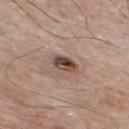<record>
<biopsy_status>not biopsied; imaged during a skin examination</biopsy_status>
<patient>
  <sex>male</sex>
  <age_approx>80</age_approx>
</patient>
<image>
  <source>total-body photography crop</source>
  <field_of_view_mm>15</field_of_view_mm>
</image>
<lighting>white-light</lighting>
<lesion_size>
  <long_diameter_mm_approx>3.5</long_diameter_mm_approx>
</lesion_size>
<site>front of the torso</site>
<automated_metrics>
  <cielab_L>46</cielab_L>
  <cielab_a>17</cielab_a>
  <cielab_b>26</cielab_b>
  <nevus_likeness_0_100>85</nevus_likeness_0_100>
  <lesion_detection_confidence_0_100>100</lesion_detection_confidence_0_100>
</automated_metrics>
</record>